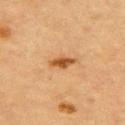Q: Was a biopsy performed?
A: no biopsy performed (imaged during a skin exam)
Q: Patient demographics?
A: female, roughly 60 years of age
Q: What is the anatomic site?
A: the upper back
Q: How was this image acquired?
A: 15 mm crop, total-body photography
Q: How large is the lesion?
A: about 3.5 mm